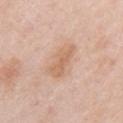No biopsy was performed on this lesion — it was imaged during a full skin examination and was not determined to be concerning.
The subject is a female in their mid- to late 60s.
From the right upper arm.
Automated tile analysis of the lesion measured a shape-asymmetry score of about 0.25 (0 = symmetric).
Imaged with white-light lighting.
Longest diameter approximately 4.5 mm.
A region of skin cropped from a whole-body photographic capture, roughly 15 mm wide.